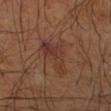follow-up: no biopsy performed (imaged during a skin exam)
tile lighting: cross-polarized
location: the left forearm
patient: male, aged 43–47
imaging modality: 15 mm crop, total-body photography
lesion size: ≈5 mm
automated metrics: a footprint of about 11 mm² and an eccentricity of roughly 0.8; a lesion color around L≈35 a*≈20 b*≈26 in CIELAB and roughly 6 lightness units darker than nearby skin; an automated nevus-likeness rating near 0 out of 100 and lesion-presence confidence of about 100/100